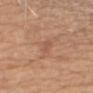{
  "biopsy_status": "not biopsied; imaged during a skin examination",
  "image": {
    "source": "total-body photography crop",
    "field_of_view_mm": 15
  },
  "lighting": "white-light",
  "automated_metrics": {
    "eccentricity": 0.9,
    "cielab_L": 54,
    "cielab_a": 22,
    "cielab_b": 31,
    "vs_skin_darker_L": 6.0,
    "vs_skin_contrast_norm": 4.5,
    "border_irregularity_0_10": 3.0,
    "color_variation_0_10": 0.0,
    "peripheral_color_asymmetry": 0.0
  },
  "patient": {
    "sex": "male",
    "age_approx": 70
  },
  "site": "right upper arm",
  "lesion_size": {
    "long_diameter_mm_approx": 2.5
  }
}